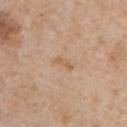Clinical impression: Recorded during total-body skin imaging; not selected for excision or biopsy. Background: A close-up tile cropped from a whole-body skin photograph, about 15 mm across. Captured under white-light illumination. The lesion's longest dimension is about 3 mm. A male subject, aged around 60. On the front of the torso.